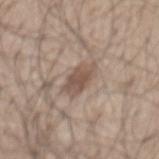<lesion>
<biopsy_status>not biopsied; imaged during a skin examination</biopsy_status>
<lesion_size>
  <long_diameter_mm_approx>4.0</long_diameter_mm_approx>
</lesion_size>
<patient>
  <sex>male</sex>
  <age_approx>50</age_approx>
</patient>
<image>
  <source>total-body photography crop</source>
  <field_of_view_mm>15</field_of_view_mm>
</image>
<lighting>white-light</lighting>
<site>back</site>
<automated_metrics>
  <vs_skin_darker_L>11.0</vs_skin_darker_L>
  <border_irregularity_0_10>2.5</border_irregularity_0_10>
  <color_variation_0_10>3.0</color_variation_0_10>
  <peripheral_color_asymmetry>1.0</peripheral_color_asymmetry>
</automated_metrics>
</lesion>